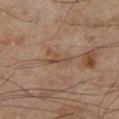| key | value |
|---|---|
| follow-up | no biopsy performed (imaged during a skin exam) |
| anatomic site | the left lower leg |
| patient | male, roughly 45 years of age |
| imaging modality | ~15 mm tile from a whole-body skin photo |